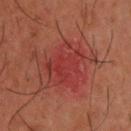Assessment:
No biopsy was performed on this lesion — it was imaged during a full skin examination and was not determined to be concerning.
Acquisition and patient details:
A roughly 15 mm field-of-view crop from a total-body skin photograph. From the upper back. Automated image analysis of the tile measured an average lesion color of about L≈38 a*≈35 b*≈27 (CIELAB), a lesion–skin lightness drop of about 6, and a normalized border contrast of about 5. The analysis additionally found a border-irregularity rating of about 5/10, a color-variation rating of about 4/10, and a peripheral color-asymmetry measure near 1.5. The recorded lesion diameter is about 5.5 mm. A male subject aged around 40. Imaged with cross-polarized lighting.